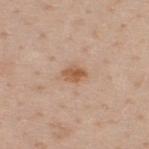Imaged during a routine full-body skin examination; the lesion was not biopsied and no histopathology is available.